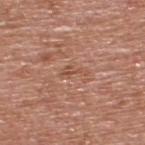This lesion was catalogued during total-body skin photography and was not selected for biopsy.
The patient is a male aged around 65.
A region of skin cropped from a whole-body photographic capture, roughly 15 mm wide.
On the upper back.